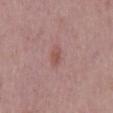notes = no biopsy performed (imaged during a skin exam) | anatomic site = the back | automated metrics = a shape eccentricity near 0.85 and a symmetry-axis asymmetry near 0.25; a border-irregularity rating of about 2.5/10, internal color variation of about 1 on a 0–10 scale, and radial color variation of about 0 | subject = male, aged approximately 60 | lesion diameter = about 2.5 mm | tile lighting = white-light | image source = ~15 mm tile from a whole-body skin photo.